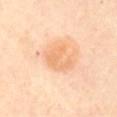This lesion was catalogued during total-body skin photography and was not selected for biopsy. A female subject, roughly 75 years of age. Captured under cross-polarized illumination. Located on the mid back. Automated image analysis of the tile measured a lesion color around L≈74 a*≈23 b*≈41 in CIELAB and a lesion-to-skin contrast of about 5 (normalized; higher = more distinct). A close-up tile cropped from a whole-body skin photograph, about 15 mm across.